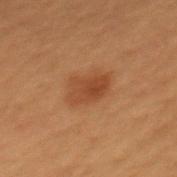Assessment:
The lesion was photographed on a routine skin check and not biopsied; there is no pathology result.
Image and clinical context:
The lesion is on the mid back. A 15 mm close-up tile from a total-body photography series done for melanoma screening. This is a cross-polarized tile. An algorithmic analysis of the crop reported an automated nevus-likeness rating near 85 out of 100 and lesion-presence confidence of about 100/100. A male subject, in their 50s.